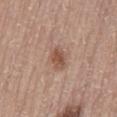workup = catalogued during a skin exam; not biopsied | acquisition = total-body-photography crop, ~15 mm field of view | lesion diameter = ≈2.5 mm | tile lighting = white-light | patient = male, aged around 55.